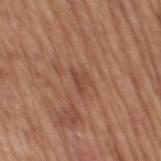{
  "biopsy_status": "not biopsied; imaged during a skin examination",
  "lesion_size": {
    "long_diameter_mm_approx": 3.0
  },
  "lighting": "white-light",
  "image": {
    "source": "total-body photography crop",
    "field_of_view_mm": 15
  },
  "patient": {
    "sex": "male",
    "age_approx": 65
  },
  "site": "mid back"
}